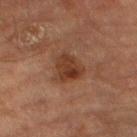* notes — no biopsy performed (imaged during a skin exam)
* tile lighting — cross-polarized
* anatomic site — the leg
* subject — male, approximately 85 years of age or older
* imaging modality — 15 mm crop, total-body photography
* lesion diameter — about 4 mm
* image-analysis metrics — an average lesion color of about L≈32 a*≈20 b*≈27 (CIELAB), roughly 8 lightness units darker than nearby skin, and a normalized border contrast of about 8.5; a border-irregularity index near 3.5/10; a classifier nevus-likeness of about 55/100 and a lesion-detection confidence of about 100/100